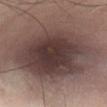This is a cross-polarized tile.
Located on the abdomen.
Cropped from a total-body skin-imaging series; the visible field is about 15 mm.
A male subject roughly 55 years of age.
Automated image analysis of the tile measured a footprint of about 70 mm² and a symmetry-axis asymmetry near 0.15. It also reported a mean CIELAB color near L≈39 a*≈15 b*≈17, about 15 CIELAB-L* units darker than the surrounding skin, and a normalized lesion–skin contrast near 12. And it measured border irregularity of about 3 on a 0–10 scale, a color-variation rating of about 6/10, and a peripheral color-asymmetry measure near 1.5.
Approximately 14 mm at its widest.